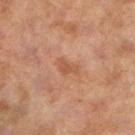No biopsy was performed on this lesion — it was imaged during a full skin examination and was not determined to be concerning.
From the left lower leg.
The patient is a female aged around 60.
This image is a 15 mm lesion crop taken from a total-body photograph.
Approximately 2.5 mm at its widest.
The tile uses cross-polarized illumination.